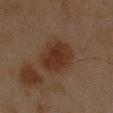Notes:
• notes: catalogued during a skin exam; not biopsied
• subject: male, aged around 45
• lighting: cross-polarized illumination
• diameter: about 5 mm
• site: the left forearm
• acquisition: total-body-photography crop, ~15 mm field of view